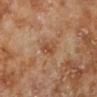Recorded during total-body skin imaging; not selected for excision or biopsy.
On the left lower leg.
This is a cross-polarized tile.
The lesion's longest dimension is about 2.5 mm.
A male subject aged 68 to 72.
Cropped from a whole-body photographic skin survey; the tile spans about 15 mm.